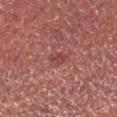biopsy_status: not biopsied; imaged during a skin examination
automated_metrics:
  nevus_likeness_0_100: 0
  lesion_detection_confidence_0_100: 100
patient:
  sex: male
  age_approx: 45
image:
  source: total-body photography crop
  field_of_view_mm: 15
site: head or neck
lesion_size:
  long_diameter_mm_approx: 3.0